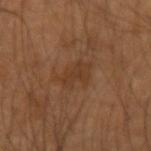Findings:
– notes · no biopsy performed (imaged during a skin exam)
– location · the arm
– acquisition · total-body-photography crop, ~15 mm field of view
– patient · male, aged around 50
– lesion size · ≈4.5 mm
– automated lesion analysis · a shape eccentricity near 0.8; a lesion color around L≈36 a*≈18 b*≈31 in CIELAB, roughly 6 lightness units darker than nearby skin, and a normalized border contrast of about 5.5; an automated nevus-likeness rating near 0 out of 100
– illumination · cross-polarized